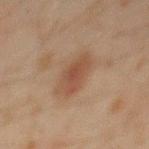Part of a total-body skin-imaging series; this lesion was reviewed on a skin check and was not flagged for biopsy.
From the mid back.
A region of skin cropped from a whole-body photographic capture, roughly 15 mm wide.
A male patient in their mid-40s.
Imaged with cross-polarized lighting.
Automated tile analysis of the lesion measured a lesion color around L≈40 a*≈16 b*≈25 in CIELAB, about 7 CIELAB-L* units darker than the surrounding skin, and a normalized lesion–skin contrast near 6.5. The analysis additionally found border irregularity of about 2 on a 0–10 scale, internal color variation of about 2.5 on a 0–10 scale, and peripheral color asymmetry of about 1.